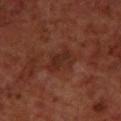<case>
<biopsy_status>not biopsied; imaged during a skin examination</biopsy_status>
<patient>
  <sex>male</sex>
  <age_approx>70</age_approx>
</patient>
<image>
  <source>total-body photography crop</source>
  <field_of_view_mm>15</field_of_view_mm>
</image>
<site>upper back</site>
</case>